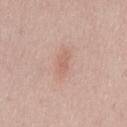Captured during whole-body skin photography for melanoma surveillance; the lesion was not biopsied. A close-up tile cropped from a whole-body skin photograph, about 15 mm across. A male patient, aged around 50. Imaged with white-light lighting. Located on the mid back. Automated image analysis of the tile measured roughly 7 lightness units darker than nearby skin and a lesion-to-skin contrast of about 4.5 (normalized; higher = more distinct). It also reported border irregularity of about 3 on a 0–10 scale and internal color variation of about 1 on a 0–10 scale.